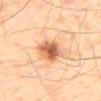No biopsy was performed on this lesion — it was imaged during a full skin examination and was not determined to be concerning.
The subject is a male aged approximately 40.
Automated image analysis of the tile measured a lesion area of about 9 mm² and a shape-asymmetry score of about 0.2 (0 = symmetric). And it measured border irregularity of about 2 on a 0–10 scale, internal color variation of about 7.5 on a 0–10 scale, and radial color variation of about 2. The analysis additionally found a nevus-likeness score of about 95/100 and lesion-presence confidence of about 100/100.
The lesion is located on the mid back.
The tile uses cross-polarized illumination.
A region of skin cropped from a whole-body photographic capture, roughly 15 mm wide.